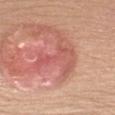This lesion was catalogued during total-body skin photography and was not selected for biopsy. A 15 mm close-up extracted from a 3D total-body photography capture. The lesion is located on the left upper arm. The total-body-photography lesion software estimated a lesion-to-skin contrast of about 5.5 (normalized; higher = more distinct). And it measured a border-irregularity index near 10/10 and radial color variation of about 1.5. The analysis additionally found an automated nevus-likeness rating near 0 out of 100 and a lesion-detection confidence of about 65/100. This is a white-light tile. A female subject aged 58 to 62.